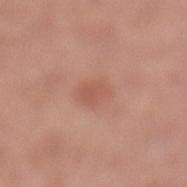No biopsy was performed on this lesion — it was imaged during a full skin examination and was not determined to be concerning. A 15 mm crop from a total-body photograph taken for skin-cancer surveillance. A female subject, aged 38–42. The lesion-visualizer software estimated an area of roughly 5 mm², an eccentricity of roughly 0.45, and two-axis asymmetry of about 0.25. It also reported a nevus-likeness score of about 20/100 and a detector confidence of about 100 out of 100 that the crop contains a lesion. Located on the left lower leg. Captured under white-light illumination.